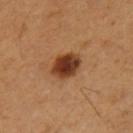biopsy status=imaged on a skin check; not biopsied
imaging modality=~15 mm crop, total-body skin-cancer survey
patient=female, in their 50s
body site=the right thigh